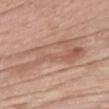Impression: Imaged during a routine full-body skin examination; the lesion was not biopsied and no histopathology is available.